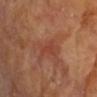Q: Patient demographics?
A: female, aged approximately 75
Q: What is the anatomic site?
A: the arm
Q: What is the imaging modality?
A: 15 mm crop, total-body photography
Q: How was the tile lit?
A: cross-polarized illumination
Q: What is the lesion's diameter?
A: ≈3 mm